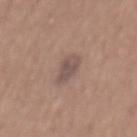Captured during whole-body skin photography for melanoma surveillance; the lesion was not biopsied. A male subject, in their mid- to late 60s. About 3.5 mm across. Captured under white-light illumination. The lesion is on the mid back. A close-up tile cropped from a whole-body skin photograph, about 15 mm across.